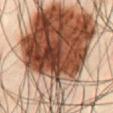biopsy status — imaged on a skin check; not biopsied | image — ~15 mm crop, total-body skin-cancer survey | site — the chest | lesion diameter — about 20.5 mm | lighting — cross-polarized illumination | image-analysis metrics — a lesion area of about 100 mm², an eccentricity of roughly 0.9, and a shape-asymmetry score of about 0.6 (0 = symmetric); a lesion color around L≈48 a*≈21 b*≈31 in CIELAB and roughly 24 lightness units darker than nearby skin; an automated nevus-likeness rating near 100 out of 100 and a lesion-detection confidence of about 95/100 | subject — male, aged 48–52.